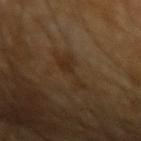Notes:
– imaging modality: ~15 mm crop, total-body skin-cancer survey
– patient: male, about 65 years old